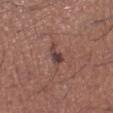Clinical impression:
The lesion was tiled from a total-body skin photograph and was not biopsied.
Acquisition and patient details:
The total-body-photography lesion software estimated an area of roughly 3.5 mm², an outline eccentricity of about 0.8 (0 = round, 1 = elongated), and a shape-asymmetry score of about 0.45 (0 = symmetric). The software also gave a lesion–skin lightness drop of about 11 and a normalized border contrast of about 9. Captured under white-light illumination. Measured at roughly 2.5 mm in maximum diameter. A close-up tile cropped from a whole-body skin photograph, about 15 mm across. From the left lower leg. A male patient in their mid-40s.